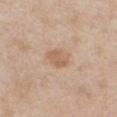Q: Was a biopsy performed?
A: imaged on a skin check; not biopsied
Q: Automated lesion metrics?
A: a footprint of about 5.5 mm², an eccentricity of roughly 0.65, and a symmetry-axis asymmetry near 0.25; a lesion color around L≈61 a*≈18 b*≈32 in CIELAB; a lesion-detection confidence of about 100/100
Q: What are the patient's age and sex?
A: female, about 40 years old
Q: Where on the body is the lesion?
A: the chest
Q: What kind of image is this?
A: ~15 mm crop, total-body skin-cancer survey